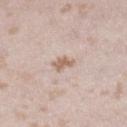* notes: total-body-photography surveillance lesion; no biopsy
* patient: female, aged around 25
* site: the right lower leg
* illumination: white-light illumination
* lesion size: about 2.5 mm
* imaging modality: ~15 mm crop, total-body skin-cancer survey